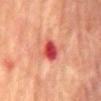  site: back
  patient:
    sex: male
    age_approx: 75
  image:
    source: total-body photography crop
    field_of_view_mm: 15